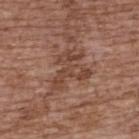biopsy status: no biopsy performed (imaged during a skin exam) | automated metrics: an outline eccentricity of about 0.6 (0 = round, 1 = elongated) and two-axis asymmetry of about 0.65; an average lesion color of about L≈44 a*≈20 b*≈28 (CIELAB), a lesion–skin lightness drop of about 8, and a lesion-to-skin contrast of about 6.5 (normalized; higher = more distinct); an automated nevus-likeness rating near 0 out of 100 and a detector confidence of about 90 out of 100 that the crop contains a lesion | illumination: white-light | patient: female, aged approximately 65 | lesion diameter: ~5 mm (longest diameter) | image source: ~15 mm crop, total-body skin-cancer survey | body site: the upper back.